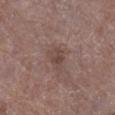<lesion>
<site>right lower leg</site>
<image>
  <source>total-body photography crop</source>
  <field_of_view_mm>15</field_of_view_mm>
</image>
<patient>
  <sex>male</sex>
  <age_approx>70</age_approx>
</patient>
<lighting>white-light</lighting>
</lesion>